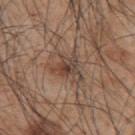The lesion was photographed on a routine skin check and not biopsied; there is no pathology result.
The patient is a male aged 43–47.
The lesion is on the upper back.
Captured under white-light illumination.
Cropped from a whole-body photographic skin survey; the tile spans about 15 mm.
The lesion-visualizer software estimated an area of roughly 8.5 mm² and an outline eccentricity of about 0.6 (0 = round, 1 = elongated). The analysis additionally found a mean CIELAB color near L≈44 a*≈16 b*≈25, about 9 CIELAB-L* units darker than the surrounding skin, and a normalized border contrast of about 7.5. The analysis additionally found a border-irregularity rating of about 6.5/10, a color-variation rating of about 6.5/10, and a peripheral color-asymmetry measure near 2. And it measured a nevus-likeness score of about 40/100 and lesion-presence confidence of about 100/100.
Measured at roughly 4.5 mm in maximum diameter.